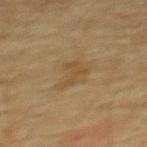- follow-up: imaged on a skin check; not biopsied
- site: the upper back
- patient: male, aged 68–72
- image source: 15 mm crop, total-body photography
- tile lighting: cross-polarized illumination
- diameter: ~3.5 mm (longest diameter)